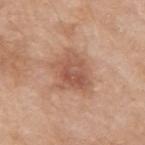acquisition=total-body-photography crop, ~15 mm field of view
size=≈5 mm
patient=female, aged around 75
location=the right upper arm
illumination=white-light
automated metrics=a lesion area of about 14 mm², an eccentricity of roughly 0.55, and two-axis asymmetry of about 0.35; roughly 10 lightness units darker than nearby skin; a color-variation rating of about 4.5/10 and radial color variation of about 1.5; a nevus-likeness score of about 75/100